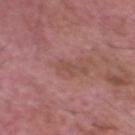Clinical impression:
No biopsy was performed on this lesion — it was imaged during a full skin examination and was not determined to be concerning.
Background:
A 15 mm crop from a total-body photograph taken for skin-cancer surveillance. The subject is a male approximately 65 years of age. This is a white-light tile. On the head or neck. Automated tile analysis of the lesion measured an area of roughly 2.5 mm², a shape eccentricity near 0.85, and a symmetry-axis asymmetry near 0.5. It also reported border irregularity of about 6 on a 0–10 scale and a peripheral color-asymmetry measure near 0. Approximately 2.5 mm at its widest.